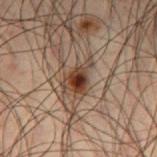biopsy status: imaged on a skin check; not biopsied
lesion size: about 4 mm
anatomic site: the left thigh
subject: male, roughly 50 years of age
lighting: cross-polarized illumination
automated lesion analysis: a lesion area of about 6.5 mm², a shape eccentricity near 0.75, and a symmetry-axis asymmetry near 0.45
acquisition: total-body-photography crop, ~15 mm field of view